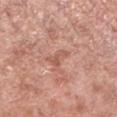Image and clinical context: A lesion tile, about 15 mm wide, cut from a 3D total-body photograph. The patient is a male roughly 55 years of age. Captured under white-light illumination. The total-body-photography lesion software estimated about 8 CIELAB-L* units darker than the surrounding skin and a normalized lesion–skin contrast near 5.5. It also reported border irregularity of about 6 on a 0–10 scale, internal color variation of about 0 on a 0–10 scale, and radial color variation of about 0. From the leg. The lesion's longest dimension is about 2.5 mm.